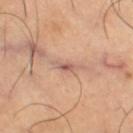This lesion was catalogued during total-body skin photography and was not selected for biopsy.
A male subject approximately 65 years of age.
A region of skin cropped from a whole-body photographic capture, roughly 15 mm wide.
Located on the right thigh.
Longest diameter approximately 3 mm.
Imaged with cross-polarized lighting.